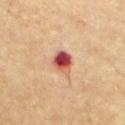Assessment: The lesion was photographed on a routine skin check and not biopsied; there is no pathology result. Context: Located on the chest. Captured under cross-polarized illumination. The lesion-visualizer software estimated an average lesion color of about L≈51 a*≈32 b*≈30 (CIELAB) and a lesion-to-skin contrast of about 12.5 (normalized; higher = more distinct). The analysis additionally found a color-variation rating of about 10/10 and a peripheral color-asymmetry measure near 3.5. A male patient, in their mid-80s or older. A 15 mm close-up tile from a total-body photography series done for melanoma screening. Approximately 3 mm at its widest.